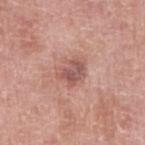Clinical summary:
The patient is a female aged approximately 60. A 15 mm crop from a total-body photograph taken for skin-cancer surveillance. The lesion is on the right upper arm.